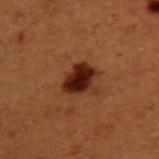Part of a total-body skin-imaging series; this lesion was reviewed on a skin check and was not flagged for biopsy.